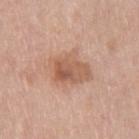The lesion was tiled from a total-body skin photograph and was not biopsied. Captured under white-light illumination. Located on the leg. Cropped from a total-body skin-imaging series; the visible field is about 15 mm. The patient is a female aged around 65. The lesion's longest dimension is about 4.5 mm.